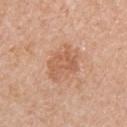| field | value |
|---|---|
| follow-up | catalogued during a skin exam; not biopsied |
| acquisition | ~15 mm crop, total-body skin-cancer survey |
| site | the right upper arm |
| subject | female, in their 70s |